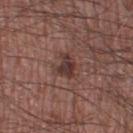Impression: Part of a total-body skin-imaging series; this lesion was reviewed on a skin check and was not flagged for biopsy. Background: A male patient aged around 60. The tile uses white-light illumination. A 15 mm close-up tile from a total-body photography series done for melanoma screening. About 3 mm across. The lesion is located on the right thigh.